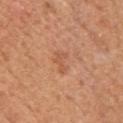{"biopsy_status": "not biopsied; imaged during a skin examination", "automated_metrics": {"area_mm2_approx": 3.0, "shape_asymmetry": 0.6}, "lighting": "white-light", "site": "front of the torso", "image": {"source": "total-body photography crop", "field_of_view_mm": 15}, "patient": {"sex": "male", "age_approx": 55}, "lesion_size": {"long_diameter_mm_approx": 2.5}}